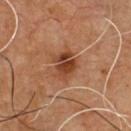Captured during whole-body skin photography for melanoma surveillance; the lesion was not biopsied.
The recorded lesion diameter is about 4 mm.
A lesion tile, about 15 mm wide, cut from a 3D total-body photograph.
Automated tile analysis of the lesion measured a footprint of about 8 mm², a shape eccentricity near 0.6, and two-axis asymmetry of about 0.25. And it measured a mean CIELAB color near L≈42 a*≈24 b*≈33 and a normalized border contrast of about 9. The software also gave border irregularity of about 3 on a 0–10 scale, a within-lesion color-variation index near 6/10, and a peripheral color-asymmetry measure near 2. The analysis additionally found a classifier nevus-likeness of about 65/100 and a detector confidence of about 100 out of 100 that the crop contains a lesion.
The lesion is on the chest.
A male patient, roughly 50 years of age.
The tile uses cross-polarized illumination.